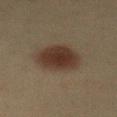<case>
<biopsy_status>not biopsied; imaged during a skin examination</biopsy_status>
<image>
  <source>total-body photography crop</source>
  <field_of_view_mm>15</field_of_view_mm>
</image>
<lesion_size>
  <long_diameter_mm_approx>5.0</long_diameter_mm_approx>
</lesion_size>
<lighting>cross-polarized</lighting>
<patient>
  <sex>female</sex>
  <age_approx>40</age_approx>
</patient>
<site>front of the torso</site>
<automated_metrics>
  <area_mm2_approx>16.0</area_mm2_approx>
  <eccentricity>0.4</eccentricity>
  <shape_asymmetry>0.15</shape_asymmetry>
  <cielab_L>29</cielab_L>
  <cielab_a>13</cielab_a>
  <cielab_b>21</cielab_b>
  <vs_skin_darker_L>10.0</vs_skin_darker_L>
  <vs_skin_contrast_norm>10.0</vs_skin_contrast_norm>
  <color_variation_0_10>3.0</color_variation_0_10>
  <peripheral_color_asymmetry>0.5</peripheral_color_asymmetry>
</automated_metrics>
</case>